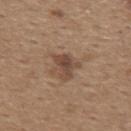Clinical impression: Recorded during total-body skin imaging; not selected for excision or biopsy. Acquisition and patient details: Longest diameter approximately 3.5 mm. The lesion is on the upper back. Imaged with white-light lighting. A male subject, aged approximately 65. A 15 mm close-up tile from a total-body photography series done for melanoma screening.